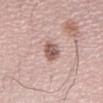| feature | finding |
|---|---|
| notes | total-body-photography surveillance lesion; no biopsy |
| tile lighting | white-light |
| subject | female, about 45 years old |
| acquisition | 15 mm crop, total-body photography |
| site | the right upper arm |
| TBP lesion metrics | border irregularity of about 2 on a 0–10 scale and a peripheral color-asymmetry measure near 1.5; a classifier nevus-likeness of about 55/100 and a lesion-detection confidence of about 100/100 |